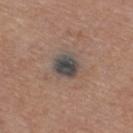• notes · no biopsy performed (imaged during a skin exam)
• lesion size · about 3.5 mm
• lighting · white-light
• patient · male, in their mid-70s
• image · total-body-photography crop, ~15 mm field of view
• body site · the leg
• TBP lesion metrics · a border-irregularity rating of about 2/10 and a within-lesion color-variation index near 5/10; a nevus-likeness score of about 0/100 and lesion-presence confidence of about 100/100